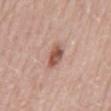Assessment:
The lesion was photographed on a routine skin check and not biopsied; there is no pathology result.
Image and clinical context:
The tile uses white-light illumination. The lesion is on the mid back. A female patient, aged 63 to 67. The lesion's longest dimension is about 3 mm. A lesion tile, about 15 mm wide, cut from a 3D total-body photograph.